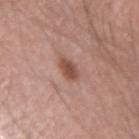Part of a total-body skin-imaging series; this lesion was reviewed on a skin check and was not flagged for biopsy.
About 2.5 mm across.
Located on the left forearm.
A 15 mm crop from a total-body photograph taken for skin-cancer surveillance.
A male subject approximately 40 years of age.
Imaged with white-light lighting.